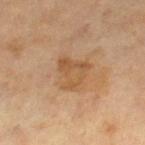Captured during whole-body skin photography for melanoma surveillance; the lesion was not biopsied. The total-body-photography lesion software estimated a border-irregularity index near 5.5/10, internal color variation of about 4 on a 0–10 scale, and radial color variation of about 1.5. And it measured a classifier nevus-likeness of about 0/100 and a lesion-detection confidence of about 100/100. The recorded lesion diameter is about 3.5 mm. Located on the right lower leg. A close-up tile cropped from a whole-body skin photograph, about 15 mm across. A female subject, aged around 55.